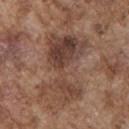{"biopsy_status": "not biopsied; imaged during a skin examination", "automated_metrics": {"cielab_L": 42, "cielab_a": 18, "cielab_b": 25, "vs_skin_darker_L": 10.0, "vs_skin_contrast_norm": 8.5, "color_variation_0_10": 7.0, "peripheral_color_asymmetry": 2.5, "nevus_likeness_0_100": 0}, "patient": {"sex": "male", "age_approx": 75}, "image": {"source": "total-body photography crop", "field_of_view_mm": 15}, "site": "chest", "lighting": "white-light", "lesion_size": {"long_diameter_mm_approx": 8.0}}